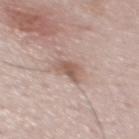site: back
lighting: white-light
image:
  source: total-body photography crop
  field_of_view_mm: 15
patient:
  sex: male
  age_approx: 45
lesion_size:
  long_diameter_mm_approx: 3.0